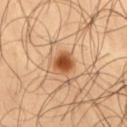The lesion is located on the right thigh.
Cropped from a whole-body photographic skin survey; the tile spans about 15 mm.
A male patient aged 53 to 57.